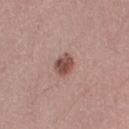Q: Is there a histopathology result?
A: catalogued during a skin exam; not biopsied
Q: What lighting was used for the tile?
A: white-light illumination
Q: Automated lesion metrics?
A: a footprint of about 4.5 mm², an outline eccentricity of about 0.65 (0 = round, 1 = elongated), and two-axis asymmetry of about 0.2; an average lesion color of about L≈49 a*≈22 b*≈24 (CIELAB), a lesion–skin lightness drop of about 14, and a lesion-to-skin contrast of about 9.5 (normalized; higher = more distinct); a border-irregularity rating of about 2/10, internal color variation of about 3.5 on a 0–10 scale, and a peripheral color-asymmetry measure near 1; a classifier nevus-likeness of about 90/100 and a detector confidence of about 100 out of 100 that the crop contains a lesion
Q: Where on the body is the lesion?
A: the right upper arm
Q: Who is the patient?
A: male, aged 38 to 42
Q: What kind of image is this?
A: total-body-photography crop, ~15 mm field of view
Q: How large is the lesion?
A: ≈2.5 mm